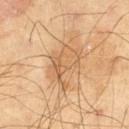Q: Was this lesion biopsied?
A: imaged on a skin check; not biopsied
Q: What is the imaging modality?
A: ~15 mm crop, total-body skin-cancer survey
Q: What is the anatomic site?
A: the right thigh
Q: Lesion size?
A: about 5 mm
Q: What are the patient's age and sex?
A: male, aged 68 to 72
Q: How was the tile lit?
A: cross-polarized